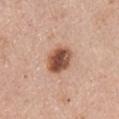workup = total-body-photography surveillance lesion; no biopsy.